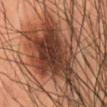Background:
A roughly 15 mm field-of-view crop from a total-body skin photograph. Imaged with cross-polarized lighting. From the lower back. The recorded lesion diameter is about 9.5 mm. The total-body-photography lesion software estimated a footprint of about 32 mm² and a symmetry-axis asymmetry near 0.35. And it measured an average lesion color of about L≈32 a*≈19 b*≈24 (CIELAB) and a normalized border contrast of about 12. A male subject in their mid-50s.